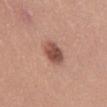| key | value |
|---|---|
| biopsy status | imaged on a skin check; not biopsied |
| illumination | white-light |
| TBP lesion metrics | a lesion area of about 6.5 mm² and two-axis asymmetry of about 0.2; an automated nevus-likeness rating near 85 out of 100 and a lesion-detection confidence of about 100/100 |
| lesion diameter | ~3.5 mm (longest diameter) |
| anatomic site | the mid back |
| subject | male, about 25 years old |
| image | ~15 mm tile from a whole-body skin photo |